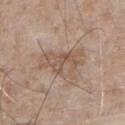workup = no biopsy performed (imaged during a skin exam) | illumination = white-light | patient = male, aged 63 to 67 | anatomic site = the chest | imaging modality = ~15 mm tile from a whole-body skin photo.